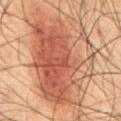The lesion was tiled from a total-body skin photograph and was not biopsied. This is a cross-polarized tile. Located on the abdomen. About 11.5 mm across. This image is a 15 mm lesion crop taken from a total-body photograph. A male patient, in their 50s.